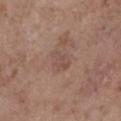biopsy status: total-body-photography surveillance lesion; no biopsy
patient: female, aged 63 to 67
automated metrics: a footprint of about 4.5 mm², a shape eccentricity near 0.8, and two-axis asymmetry of about 0.3; an average lesion color of about L≈49 a*≈18 b*≈23 (CIELAB), about 6 CIELAB-L* units darker than the surrounding skin, and a normalized lesion–skin contrast near 4.5; border irregularity of about 3 on a 0–10 scale, a color-variation rating of about 3.5/10, and a peripheral color-asymmetry measure near 1.5; an automated nevus-likeness rating near 0 out of 100 and lesion-presence confidence of about 100/100
image: ~15 mm crop, total-body skin-cancer survey
body site: the right lower leg